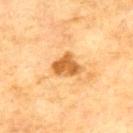The lesion was tiled from a total-body skin photograph and was not biopsied.
The lesion's longest dimension is about 3.5 mm.
The lesion is on the back.
A male subject about 70 years old.
This image is a 15 mm lesion crop taken from a total-body photograph.
This is a cross-polarized tile.